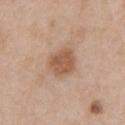Q: Is there a histopathology result?
A: catalogued during a skin exam; not biopsied
Q: Lesion size?
A: ~3.5 mm (longest diameter)
Q: Illumination type?
A: white-light illumination
Q: How was this image acquired?
A: ~15 mm crop, total-body skin-cancer survey
Q: Where on the body is the lesion?
A: the chest
Q: Who is the patient?
A: male, about 60 years old
Q: What did automated image analysis measure?
A: a lesion area of about 9.5 mm² and a symmetry-axis asymmetry near 0.2; a lesion color around L≈55 a*≈19 b*≈31 in CIELAB, about 11 CIELAB-L* units darker than the surrounding skin, and a normalized lesion–skin contrast near 7.5; a nevus-likeness score of about 65/100 and lesion-presence confidence of about 100/100